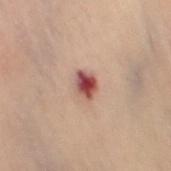lesion size: ≈2.5 mm; automated lesion analysis: a border-irregularity rating of about 2.5/10, a within-lesion color-variation index near 7/10, and a peripheral color-asymmetry measure near 2; subject: female, roughly 60 years of age; illumination: cross-polarized; imaging modality: ~15 mm tile from a whole-body skin photo; location: the lower back.